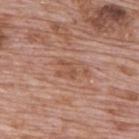No biopsy was performed on this lesion — it was imaged during a full skin examination and was not determined to be concerning. Cropped from a total-body skin-imaging series; the visible field is about 15 mm. Longest diameter approximately 2.5 mm. A male patient, aged 68 to 72. This is a white-light tile. The lesion is on the upper back. Automated tile analysis of the lesion measured a footprint of about 2 mm² and two-axis asymmetry of about 0.55. It also reported a within-lesion color-variation index near 0/10 and radial color variation of about 0.